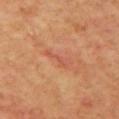A 15 mm crop from a total-body photograph taken for skin-cancer surveillance.
The recorded lesion diameter is about 3.5 mm.
A female patient roughly 40 years of age.
Located on the chest.
Automated tile analysis of the lesion measured a lesion area of about 4 mm², an outline eccentricity of about 0.95 (0 = round, 1 = elongated), and a symmetry-axis asymmetry near 0.5. The software also gave a classifier nevus-likeness of about 0/100.